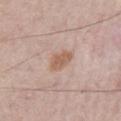Q: Is there a histopathology result?
A: catalogued during a skin exam; not biopsied